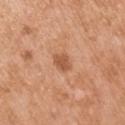Q: Was a biopsy performed?
A: no biopsy performed (imaged during a skin exam)
Q: What is the anatomic site?
A: the arm
Q: What is the imaging modality?
A: ~15 mm tile from a whole-body skin photo
Q: Lesion size?
A: ≈2.5 mm
Q: What did automated image analysis measure?
A: a lesion color around L≈55 a*≈25 b*≈35 in CIELAB, roughly 11 lightness units darker than nearby skin, and a normalized lesion–skin contrast near 7
Q: Who is the patient?
A: male, aged 53–57